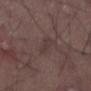<lesion>
<lesion_size>
  <long_diameter_mm_approx>3.0</long_diameter_mm_approx>
</lesion_size>
<site>lower back</site>
<image>
  <source>total-body photography crop</source>
  <field_of_view_mm>15</field_of_view_mm>
</image>
<lighting>white-light</lighting>
<patient>
  <sex>male</sex>
  <age_approx>50</age_approx>
</patient>
<automated_metrics>
  <area_mm2_approx>3.5</area_mm2_approx>
  <eccentricity>0.95</eccentricity>
  <cielab_L>36</cielab_L>
  <cielab_a>15</cielab_a>
  <cielab_b>16</cielab_b>
  <vs_skin_darker_L>5.0</vs_skin_darker_L>
  <vs_skin_contrast_norm>4.5</vs_skin_contrast_norm>
  <border_irregularity_0_10>3.5</border_irregularity_0_10>
  <color_variation_0_10>0.0</color_variation_0_10>
  <peripheral_color_asymmetry>0.0</peripheral_color_asymmetry>
  <lesion_detection_confidence_0_100>65</lesion_detection_confidence_0_100>
</automated_metrics>
</lesion>